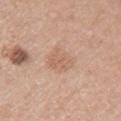Recorded during total-body skin imaging; not selected for excision or biopsy. Located on the arm. Approximately 3.5 mm at its widest. Captured under white-light illumination. A female patient about 55 years old. A 15 mm crop from a total-body photograph taken for skin-cancer surveillance.